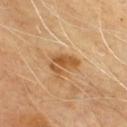Clinical impression:
Recorded during total-body skin imaging; not selected for excision or biopsy.
Context:
A roughly 15 mm field-of-view crop from a total-body skin photograph. The subject is a male in their mid-60s. The recorded lesion diameter is about 3.5 mm. Imaged with cross-polarized lighting. Located on the chest.